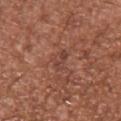{"site": "chest", "image": {"source": "total-body photography crop", "field_of_view_mm": 15}, "lighting": "white-light", "patient": {"sex": "male", "age_approx": 45}, "lesion_size": {"long_diameter_mm_approx": 3.0}}